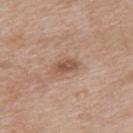| key | value |
|---|---|
| workup | catalogued during a skin exam; not biopsied |
| imaging modality | 15 mm crop, total-body photography |
| subject | female, approximately 40 years of age |
| anatomic site | the upper back |